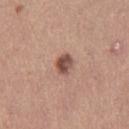This lesion was catalogued during total-body skin photography and was not selected for biopsy.
Located on the right thigh.
Measured at roughly 2.5 mm in maximum diameter.
Captured under white-light illumination.
A close-up tile cropped from a whole-body skin photograph, about 15 mm across.
The subject is a female in their mid- to late 30s.